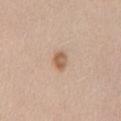{"biopsy_status": "not biopsied; imaged during a skin examination", "image": {"source": "total-body photography crop", "field_of_view_mm": 15}, "lesion_size": {"long_diameter_mm_approx": 2.5}, "automated_metrics": {"cielab_L": 60, "cielab_a": 18, "cielab_b": 31, "vs_skin_darker_L": 11.0, "color_variation_0_10": 2.5, "peripheral_color_asymmetry": 1.0}, "site": "mid back", "patient": {"sex": "female", "age_approx": 50}, "lighting": "white-light"}